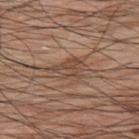The lesion was tiled from a total-body skin photograph and was not biopsied. About 3.5 mm across. The lesion is located on the upper back. The subject is a male aged 68 to 72. This is a white-light tile. A 15 mm crop from a total-body photograph taken for skin-cancer surveillance.